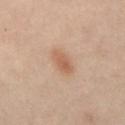Captured during whole-body skin photography for melanoma surveillance; the lesion was not biopsied.
A close-up tile cropped from a whole-body skin photograph, about 15 mm across.
A male subject, aged 48 to 52.
From the abdomen.
The recorded lesion diameter is about 3 mm.